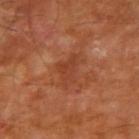No biopsy was performed on this lesion — it was imaged during a full skin examination and was not determined to be concerning.
This is a cross-polarized tile.
The patient is a male aged 68–72.
The recorded lesion diameter is about 4 mm.
From the arm.
Cropped from a whole-body photographic skin survey; the tile spans about 15 mm.